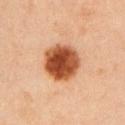lesion_size:
  long_diameter_mm_approx: 5.0
patient:
  sex: female
  age_approx: 45
image:
  source: total-body photography crop
  field_of_view_mm: 15
site: left upper arm
lighting: cross-polarized